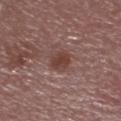Imaged during a routine full-body skin examination; the lesion was not biopsied and no histopathology is available. The subject is a male aged around 55. Approximately 3 mm at its widest. The lesion is located on the head or neck. A 15 mm crop from a total-body photograph taken for skin-cancer surveillance. Captured under white-light illumination.